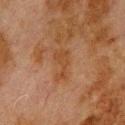Notes:
* follow-up · no biopsy performed (imaged during a skin exam)
* subject · male, about 80 years old
* size · about 3.5 mm
* TBP lesion metrics · a footprint of about 4.5 mm², an outline eccentricity of about 0.85 (0 = round, 1 = elongated), and a symmetry-axis asymmetry near 0.6; a classifier nevus-likeness of about 0/100
* acquisition · total-body-photography crop, ~15 mm field of view
* illumination · cross-polarized
* location · the upper back